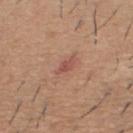Part of a total-body skin-imaging series; this lesion was reviewed on a skin check and was not flagged for biopsy. Imaged with white-light lighting. Cropped from a whole-body photographic skin survey; the tile spans about 15 mm. The lesion's longest dimension is about 2.5 mm. The patient is a male aged approximately 60. Located on the upper back.